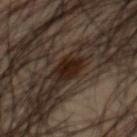Assessment: Part of a total-body skin-imaging series; this lesion was reviewed on a skin check and was not flagged for biopsy. Image and clinical context: The lesion is on the chest. A male patient aged 43–47. Cropped from a whole-body photographic skin survey; the tile spans about 15 mm. The lesion's longest dimension is about 3 mm. Imaged with cross-polarized lighting.